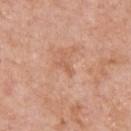| field | value |
|---|---|
| biopsy status | total-body-photography surveillance lesion; no biopsy |